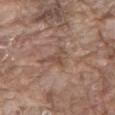The lesion was tiled from a total-body skin photograph and was not biopsied.
The patient is a male aged around 80.
A roughly 15 mm field-of-view crop from a total-body skin photograph.
The lesion is located on the mid back.